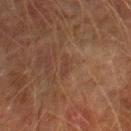Impression: Recorded during total-body skin imaging; not selected for excision or biopsy. Background: On the right lower leg. Captured under cross-polarized illumination. A male subject in their mid- to late 70s. Cropped from a total-body skin-imaging series; the visible field is about 15 mm.